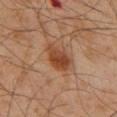{"biopsy_status": "not biopsied; imaged during a skin examination", "automated_metrics": {"cielab_L": 40, "cielab_a": 21, "cielab_b": 31, "vs_skin_darker_L": 10.0, "vs_skin_contrast_norm": 9.0}, "site": "chest", "lighting": "cross-polarized", "lesion_size": {"long_diameter_mm_approx": 4.0}, "patient": {"sex": "male", "age_approx": 60}, "image": {"source": "total-body photography crop", "field_of_view_mm": 15}}